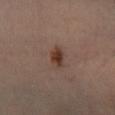The lesion was tiled from a total-body skin photograph and was not biopsied. A male patient about 55 years old. A 15 mm crop from a total-body photograph taken for skin-cancer surveillance. The lesion is on the leg. Automated image analysis of the tile measured a lesion area of about 4.5 mm², an outline eccentricity of about 0.6 (0 = round, 1 = elongated), and two-axis asymmetry of about 0.2. The analysis additionally found a mean CIELAB color near L≈37 a*≈18 b*≈25 and a normalized border contrast of about 9. The software also gave a border-irregularity rating of about 2/10, a within-lesion color-variation index near 3.5/10, and a peripheral color-asymmetry measure near 1. Imaged with cross-polarized lighting. Approximately 2.5 mm at its widest.